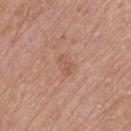Case summary:
• workup · imaged on a skin check; not biopsied
• imaging modality · ~15 mm crop, total-body skin-cancer survey
• lighting · white-light illumination
• location · the upper back
• TBP lesion metrics · a shape-asymmetry score of about 0.45 (0 = symmetric); an automated nevus-likeness rating near 0 out of 100 and lesion-presence confidence of about 100/100
• subject · male, aged around 75
• size · ≈2.5 mm